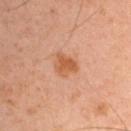follow-up = total-body-photography surveillance lesion; no biopsy
TBP lesion metrics = a lesion color around L≈54 a*≈24 b*≈36 in CIELAB, roughly 9 lightness units darker than nearby skin, and a normalized lesion–skin contrast near 7.5; a classifier nevus-likeness of about 80/100
subject = male, aged 43–47
size = ~3 mm (longest diameter)
illumination = cross-polarized
acquisition = ~15 mm tile from a whole-body skin photo
site = the left upper arm